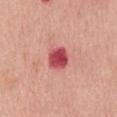subject: male, about 70 years old
image: 15 mm crop, total-body photography
tile lighting: white-light illumination
image-analysis metrics: a lesion area of about 6.5 mm² and two-axis asymmetry of about 0.15; an average lesion color of about L≈51 a*≈40 b*≈24 (CIELAB) and a lesion-to-skin contrast of about 11 (normalized; higher = more distinct); border irregularity of about 1 on a 0–10 scale, a color-variation rating of about 4/10, and radial color variation of about 1.5
location: the abdomen